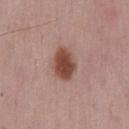The lesion was tiled from a total-body skin photograph and was not biopsied. On the back. Captured under white-light illumination. A roughly 15 mm field-of-view crop from a total-body skin photograph. Longest diameter approximately 4 mm. A male patient aged around 50.